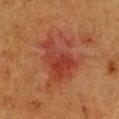| key | value |
|---|---|
| workup | catalogued during a skin exam; not biopsied |
| image-analysis metrics | a mean CIELAB color near L≈35 a*≈25 b*≈28, a lesion–skin lightness drop of about 7, and a normalized border contrast of about 6.5 |
| site | the chest |
| lesion size | about 7 mm |
| tile lighting | cross-polarized |
| acquisition | ~15 mm crop, total-body skin-cancer survey |
| patient | female, about 40 years old |